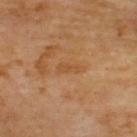Notes:
* acquisition — ~15 mm crop, total-body skin-cancer survey
* body site — the upper back
* patient — male, aged 68–72
* automated lesion analysis — a lesion area of about 3.5 mm², a shape eccentricity near 0.95, and a shape-asymmetry score of about 0.35 (0 = symmetric)